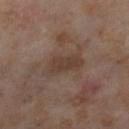The lesion was tiled from a total-body skin photograph and was not biopsied.
A roughly 15 mm field-of-view crop from a total-body skin photograph.
The tile uses cross-polarized illumination.
The lesion is located on the right lower leg.
A female subject roughly 55 years of age.